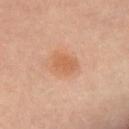| key | value |
|---|---|
| follow-up | catalogued during a skin exam; not biopsied |
| image-analysis metrics | a footprint of about 6 mm², an eccentricity of roughly 0.6, and a symmetry-axis asymmetry near 0.15; an average lesion color of about L≈58 a*≈23 b*≈35 (CIELAB) and about 8 CIELAB-L* units darker than the surrounding skin; a classifier nevus-likeness of about 60/100 and a lesion-detection confidence of about 100/100 |
| patient | female, aged around 50 |
| lighting | cross-polarized illumination |
| image | ~15 mm crop, total-body skin-cancer survey |
| body site | the upper back |
| size | ~3 mm (longest diameter) |